A region of skin cropped from a whole-body photographic capture, roughly 15 mm wide. This is a cross-polarized tile. Automated image analysis of the tile measured a footprint of about 16 mm², an eccentricity of roughly 0.3, and two-axis asymmetry of about 0.15. Measured at roughly 4.5 mm in maximum diameter. The subject is a female aged 53 to 57. From the chest — 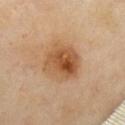On excision, pathology confirmed a lesion of indeterminate malignant potential: atypical melanocytic neoplasm.